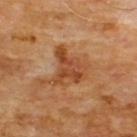{
  "biopsy_status": "not biopsied; imaged during a skin examination",
  "site": "chest",
  "lesion_size": {
    "long_diameter_mm_approx": 4.5
  },
  "lighting": "cross-polarized",
  "image": {
    "source": "total-body photography crop",
    "field_of_view_mm": 15
  },
  "patient": {
    "sex": "male",
    "age_approx": 60
  }
}